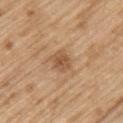The lesion was tiled from a total-body skin photograph and was not biopsied. Imaged with white-light lighting. A roughly 15 mm field-of-view crop from a total-body skin photograph. About 2.5 mm across. Automated tile analysis of the lesion measured roughly 9 lightness units darker than nearby skin and a normalized lesion–skin contrast near 6.5. And it measured a border-irregularity index near 2.5/10, a color-variation rating of about 4/10, and peripheral color asymmetry of about 1.5. The analysis additionally found a nevus-likeness score of about 35/100 and a detector confidence of about 100 out of 100 that the crop contains a lesion. The subject is a male aged 68–72. From the left upper arm.